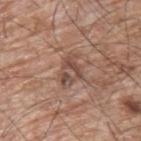Q: Was a biopsy performed?
A: total-body-photography surveillance lesion; no biopsy
Q: How was the tile lit?
A: white-light
Q: What kind of image is this?
A: ~15 mm crop, total-body skin-cancer survey
Q: Lesion size?
A: ≈4 mm
Q: Who is the patient?
A: male, aged approximately 65
Q: Where on the body is the lesion?
A: the upper back